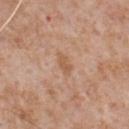Case summary:
• notes: catalogued during a skin exam; not biopsied
• subject: male, in their mid- to late 60s
• image: ~15 mm tile from a whole-body skin photo
• tile lighting: white-light illumination
• site: the chest